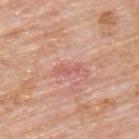Impression: Captured during whole-body skin photography for melanoma surveillance; the lesion was not biopsied. Background: The patient is a male aged approximately 75. Cropped from a total-body skin-imaging series; the visible field is about 15 mm. The tile uses white-light illumination. From the upper back.